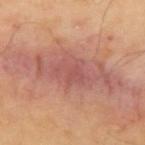{"biopsy_status": "not biopsied; imaged during a skin examination"}